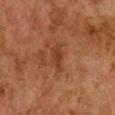Q: Was a biopsy performed?
A: no biopsy performed (imaged during a skin exam)
Q: What is the anatomic site?
A: the chest
Q: Automated lesion metrics?
A: a lesion area of about 4 mm² and a shape-asymmetry score of about 0.25 (0 = symmetric)
Q: How was the tile lit?
A: cross-polarized illumination
Q: How was this image acquired?
A: ~15 mm tile from a whole-body skin photo
Q: How large is the lesion?
A: about 3 mm
Q: What are the patient's age and sex?
A: female, about 60 years old